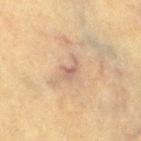Q: Was this lesion biopsied?
A: catalogued during a skin exam; not biopsied
Q: How was this image acquired?
A: ~15 mm crop, total-body skin-cancer survey
Q: Patient demographics?
A: female, about 55 years old
Q: Where on the body is the lesion?
A: the right thigh
Q: Automated lesion metrics?
A: a border-irregularity rating of about 5.5/10, a within-lesion color-variation index near 2/10, and peripheral color asymmetry of about 0.5
Q: How was the tile lit?
A: cross-polarized
Q: What is the lesion's diameter?
A: ~2.5 mm (longest diameter)